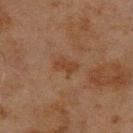Findings:
– workup · catalogued during a skin exam; not biopsied